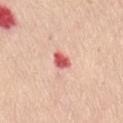- follow-up · total-body-photography surveillance lesion; no biopsy
- location · the abdomen
- lesion diameter · about 2.5 mm
- lighting · white-light illumination
- image · ~15 mm crop, total-body skin-cancer survey
- subject · female, in their mid- to late 40s
- image-analysis metrics · a mean CIELAB color near L≈60 a*≈37 b*≈27 and a normalized border contrast of about 10; a border-irregularity index near 2/10 and peripheral color asymmetry of about 1; a classifier nevus-likeness of about 0/100 and lesion-presence confidence of about 100/100